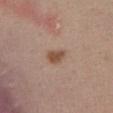biopsy status: total-body-photography surveillance lesion; no biopsy.